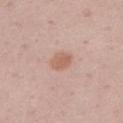Recorded during total-body skin imaging; not selected for excision or biopsy. The lesion is located on the left upper arm. Imaged with white-light lighting. A lesion tile, about 15 mm wide, cut from a 3D total-body photograph. A male patient approximately 50 years of age. About 3 mm across.